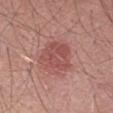biopsy status: catalogued during a skin exam; not biopsied | body site: the arm | lesion diameter: about 4 mm | illumination: white-light | image: ~15 mm tile from a whole-body skin photo | patient: male, aged 48 to 52.